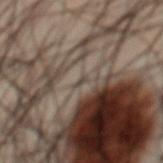| feature | finding |
|---|---|
| subject | male, in their 50s |
| lesion size | about 1.5 mm |
| imaging modality | ~15 mm crop, total-body skin-cancer survey |
| illumination | cross-polarized |
| body site | the chest |